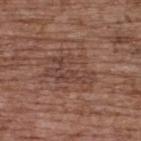Q: Is there a histopathology result?
A: catalogued during a skin exam; not biopsied